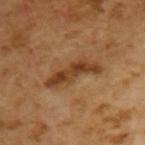Q: Was a biopsy performed?
A: catalogued during a skin exam; not biopsied
Q: What is the lesion's diameter?
A: about 6 mm
Q: How was the tile lit?
A: cross-polarized
Q: Patient demographics?
A: male, aged 63–67
Q: What kind of image is this?
A: ~15 mm crop, total-body skin-cancer survey